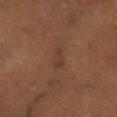The lesion was tiled from a total-body skin photograph and was not biopsied.
A male patient approximately 50 years of age.
On the right lower leg.
A lesion tile, about 15 mm wide, cut from a 3D total-body photograph.
About 3 mm across.